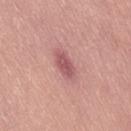{"biopsy_status": "not biopsied; imaged during a skin examination", "site": "right thigh", "patient": {"sex": "male", "age_approx": 40}, "image": {"source": "total-body photography crop", "field_of_view_mm": 15}, "automated_metrics": {"vs_skin_contrast_norm": 8.0, "border_irregularity_0_10": 2.0, "color_variation_0_10": 1.5, "peripheral_color_asymmetry": 0.5, "nevus_likeness_0_100": 10, "lesion_detection_confidence_0_100": 100}, "lighting": "white-light"}